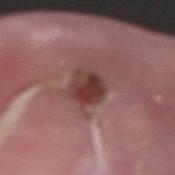Clinical impression: Imaged during a routine full-body skin examination; the lesion was not biopsied and no histopathology is available. Background: The lesion's longest dimension is about 4 mm. This is a white-light tile. The lesion-visualizer software estimated a lesion area of about 9 mm², an outline eccentricity of about 0.55 (0 = round, 1 = elongated), and a symmetry-axis asymmetry near 0.2. And it measured about 12 CIELAB-L* units darker than the surrounding skin. The analysis additionally found a border-irregularity rating of about 2/10 and a within-lesion color-variation index near 7/10. The analysis additionally found a detector confidence of about 100 out of 100 that the crop contains a lesion. On the arm. A 15 mm crop from a total-body photograph taken for skin-cancer surveillance. A male subject, about 45 years old.